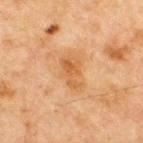Assessment:
Captured during whole-body skin photography for melanoma surveillance; the lesion was not biopsied.
Image and clinical context:
This is a cross-polarized tile. The recorded lesion diameter is about 4 mm. The lesion-visualizer software estimated an eccentricity of roughly 0.85. The software also gave border irregularity of about 4.5 on a 0–10 scale, a within-lesion color-variation index near 3.5/10, and a peripheral color-asymmetry measure near 1. The software also gave a nevus-likeness score of about 5/100 and lesion-presence confidence of about 100/100. The subject is a male aged around 65. A roughly 15 mm field-of-view crop from a total-body skin photograph. Located on the chest.